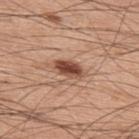Q: Was a biopsy performed?
A: catalogued during a skin exam; not biopsied
Q: How large is the lesion?
A: about 3.5 mm
Q: How was this image acquired?
A: 15 mm crop, total-body photography
Q: Where on the body is the lesion?
A: the back
Q: Illumination type?
A: white-light illumination
Q: What are the patient's age and sex?
A: male, aged around 60